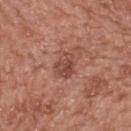biopsy status: no biopsy performed (imaged during a skin exam); acquisition: total-body-photography crop, ~15 mm field of view; lesion size: about 3 mm; patient: female, aged 58–62; site: the upper back.